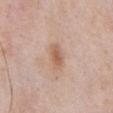Acquisition and patient details:
Cropped from a total-body skin-imaging series; the visible field is about 15 mm. From the abdomen. A male subject aged 53 to 57. Longest diameter approximately 3.5 mm. This is a white-light tile.